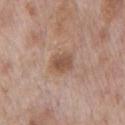Notes:
– image source — ~15 mm tile from a whole-body skin photo
– location — the chest
– patient — male, aged 68 to 72
– lesion size — ~3 mm (longest diameter)